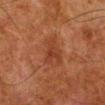biopsy status = imaged on a skin check; not biopsied
acquisition = ~15 mm crop, total-body skin-cancer survey
tile lighting = cross-polarized
subject = male, aged 78–82
anatomic site = the right lower leg
lesion diameter = about 4 mm
image-analysis metrics = an area of roughly 9.5 mm²; a mean CIELAB color near L≈32 a*≈22 b*≈28 and a normalized border contrast of about 5.5; a border-irregularity index near 3/10, a color-variation rating of about 3/10, and a peripheral color-asymmetry measure near 1